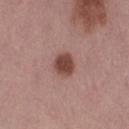Q: Was this lesion biopsied?
A: imaged on a skin check; not biopsied
Q: Who is the patient?
A: female, aged approximately 50
Q: What is the anatomic site?
A: the right thigh
Q: What kind of image is this?
A: 15 mm crop, total-body photography